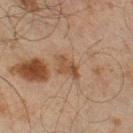Recorded during total-body skin imaging; not selected for excision or biopsy. Imaged with cross-polarized lighting. Located on the right lower leg. A 15 mm close-up extracted from a 3D total-body photography capture. The subject is a male aged approximately 45. The recorded lesion diameter is about 4 mm.